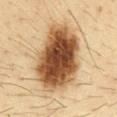Imaged during a routine full-body skin examination; the lesion was not biopsied and no histopathology is available.